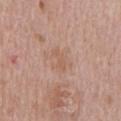No biopsy was performed on this lesion — it was imaged during a full skin examination and was not determined to be concerning. Longest diameter approximately 3 mm. On the chest. An algorithmic analysis of the crop reported a lesion area of about 3 mm² and two-axis asymmetry of about 0.45. The analysis additionally found an average lesion color of about L≈58 a*≈19 b*≈29 (CIELAB), roughly 6 lightness units darker than nearby skin, and a normalized lesion–skin contrast near 5. The software also gave border irregularity of about 5 on a 0–10 scale and a peripheral color-asymmetry measure near 0. The analysis additionally found a nevus-likeness score of about 0/100 and a lesion-detection confidence of about 100/100. Cropped from a whole-body photographic skin survey; the tile spans about 15 mm. A male subject about 70 years old.